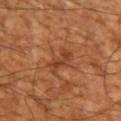Part of a total-body skin-imaging series; this lesion was reviewed on a skin check and was not flagged for biopsy. A subject in their mid-60s. The lesion is located on the left upper arm. This image is a 15 mm lesion crop taken from a total-body photograph. The total-body-photography lesion software estimated a footprint of about 6 mm². This is a cross-polarized tile. The lesion's longest dimension is about 4 mm.